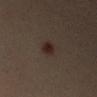Impression:
No biopsy was performed on this lesion — it was imaged during a full skin examination and was not determined to be concerning.
Clinical summary:
On the right upper arm. A male subject, roughly 40 years of age. A lesion tile, about 15 mm wide, cut from a 3D total-body photograph. The total-body-photography lesion software estimated a footprint of about 4.5 mm², an outline eccentricity of about 0.75 (0 = round, 1 = elongated), and two-axis asymmetry of about 0.2. It also reported a mean CIELAB color near L≈21 a*≈12 b*≈16, about 6 CIELAB-L* units darker than the surrounding skin, and a lesion-to-skin contrast of about 8.5 (normalized; higher = more distinct). Longest diameter approximately 3 mm.